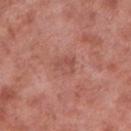A region of skin cropped from a whole-body photographic capture, roughly 15 mm wide.
Approximately 2.5 mm at its widest.
The lesion is on the right lower leg.
A male subject, aged 53 to 57.
Captured under white-light illumination.